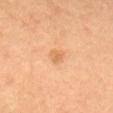Findings:
* biopsy status — imaged on a skin check; not biopsied
* patient — female, aged 28–32
* diameter — about 2 mm
* tile lighting — cross-polarized illumination
* anatomic site — the head or neck
* image — total-body-photography crop, ~15 mm field of view